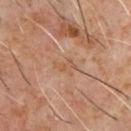Notes:
* notes: no biopsy performed (imaged during a skin exam)
* diameter: ≈2.5 mm
* patient: male, roughly 60 years of age
* location: the chest
* image: ~15 mm tile from a whole-body skin photo
* lighting: cross-polarized illumination